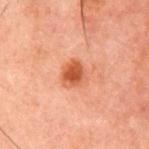The lesion was photographed on a routine skin check and not biopsied; there is no pathology result. From the chest. Cropped from a total-body skin-imaging series; the visible field is about 15 mm. A male subject in their 60s. The lesion's longest dimension is about 2.5 mm.